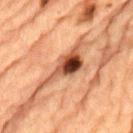Q: Is there a histopathology result?
A: total-body-photography surveillance lesion; no biopsy
Q: What is the lesion's diameter?
A: ≈7 mm
Q: What is the anatomic site?
A: the lower back
Q: Patient demographics?
A: male, about 85 years old or older
Q: How was this image acquired?
A: ~15 mm tile from a whole-body skin photo
Q: How was the tile lit?
A: cross-polarized